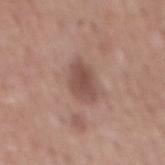Q: What kind of image is this?
A: 15 mm crop, total-body photography
Q: What lighting was used for the tile?
A: white-light illumination
Q: Lesion size?
A: about 4 mm
Q: Patient demographics?
A: male, aged 53–57
Q: Where on the body is the lesion?
A: the mid back